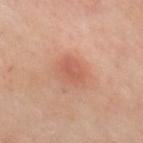Assessment:
Recorded during total-body skin imaging; not selected for excision or biopsy.
Background:
The lesion's longest dimension is about 3 mm. A region of skin cropped from a whole-body photographic capture, roughly 15 mm wide. An algorithmic analysis of the crop reported a mean CIELAB color near L≈59 a*≈27 b*≈33 and a lesion–skin lightness drop of about 8. The analysis additionally found border irregularity of about 2 on a 0–10 scale, internal color variation of about 3 on a 0–10 scale, and radial color variation of about 1. The lesion is on the back. A female subject in their mid- to late 50s.